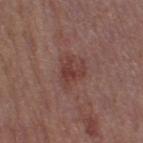Findings:
- biopsy status · imaged on a skin check; not biopsied
- illumination · white-light illumination
- patient · female, aged approximately 40
- site · the right thigh
- lesion diameter · ≈4 mm
- image source · ~15 mm tile from a whole-body skin photo